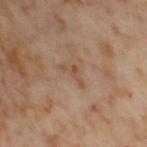The lesion was photographed on a routine skin check and not biopsied; there is no pathology result.
On the leg.
The subject is a female approximately 55 years of age.
This image is a 15 mm lesion crop taken from a total-body photograph.